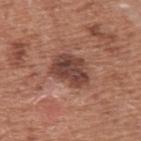Part of a total-body skin-imaging series; this lesion was reviewed on a skin check and was not flagged for biopsy. A 15 mm close-up tile from a total-body photography series done for melanoma screening. Approximately 4.5 mm at its widest. The lesion-visualizer software estimated a border-irregularity index near 2.5/10 and a color-variation rating of about 4.5/10. It also reported an automated nevus-likeness rating near 5 out of 100 and a lesion-detection confidence of about 100/100. The lesion is on the upper back. A male patient in their mid-70s.